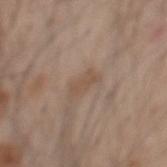notes: no biopsy performed (imaged during a skin exam); imaging modality: 15 mm crop, total-body photography; lighting: white-light illumination; patient: male, aged approximately 60; anatomic site: the mid back; size: ~3 mm (longest diameter); TBP lesion metrics: a lesion area of about 3.5 mm², an outline eccentricity of about 0.9 (0 = round, 1 = elongated), and two-axis asymmetry of about 0.4.